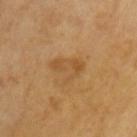workup: total-body-photography surveillance lesion; no biopsy
patient: male, aged approximately 65
acquisition: 15 mm crop, total-body photography
body site: the chest
automated lesion analysis: a footprint of about 6.5 mm² and an outline eccentricity of about 0.75 (0 = round, 1 = elongated); border irregularity of about 7 on a 0–10 scale and radial color variation of about 1; lesion-presence confidence of about 100/100
illumination: cross-polarized
lesion size: about 4 mm